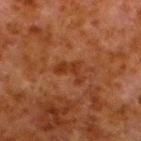Case summary:
– notes: imaged on a skin check; not biopsied
– patient: male, aged approximately 80
– lesion diameter: ≈3.5 mm
– location: the left lower leg
– imaging modality: total-body-photography crop, ~15 mm field of view
– automated metrics: a symmetry-axis asymmetry near 0.75; a classifier nevus-likeness of about 0/100 and lesion-presence confidence of about 100/100